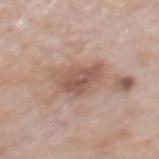Recorded during total-body skin imaging; not selected for excision or biopsy. A roughly 15 mm field-of-view crop from a total-body skin photograph. The lesion's longest dimension is about 4 mm. The tile uses white-light illumination. From the mid back. A male patient, in their mid- to late 70s.